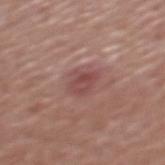Imaged during a routine full-body skin examination; the lesion was not biopsied and no histopathology is available. The subject is a male in their mid-70s. The lesion is on the mid back. A 15 mm close-up extracted from a 3D total-body photography capture.